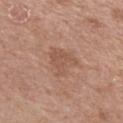Impression:
Part of a total-body skin-imaging series; this lesion was reviewed on a skin check and was not flagged for biopsy.
Clinical summary:
A female patient, approximately 40 years of age. A region of skin cropped from a whole-body photographic capture, roughly 15 mm wide. Automated tile analysis of the lesion measured a footprint of about 9 mm². And it measured a mean CIELAB color near L≈53 a*≈20 b*≈29, a lesion–skin lightness drop of about 7, and a normalized lesion–skin contrast near 5. Captured under white-light illumination. The recorded lesion diameter is about 4 mm. Located on the left upper arm.